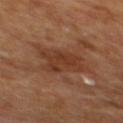Assessment: Captured during whole-body skin photography for melanoma surveillance; the lesion was not biopsied. Clinical summary: A male patient aged 63 to 67. The lesion is located on the mid back. Captured under cross-polarized illumination. Cropped from a whole-body photographic skin survey; the tile spans about 15 mm.